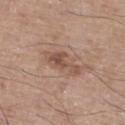Captured during whole-body skin photography for melanoma surveillance; the lesion was not biopsied. Captured under white-light illumination. Approximately 4.5 mm at its widest. Automated tile analysis of the lesion measured a border-irregularity index near 5.5/10 and a color-variation rating of about 3.5/10. Cropped from a total-body skin-imaging series; the visible field is about 15 mm. A male subject, aged 63 to 67. From the right lower leg.